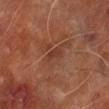Clinical impression:
Imaged during a routine full-body skin examination; the lesion was not biopsied and no histopathology is available.
Acquisition and patient details:
About 2.5 mm across. Located on the right lower leg. A male subject about 70 years old. Cropped from a total-body skin-imaging series; the visible field is about 15 mm.